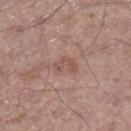Automated image analysis of the tile measured a lesion area of about 4 mm², an eccentricity of roughly 0.8, and two-axis asymmetry of about 0.4. It also reported a border-irregularity index near 5/10, a color-variation rating of about 0.5/10, and peripheral color asymmetry of about 0. It also reported a nevus-likeness score of about 0/100 and lesion-presence confidence of about 100/100. A male patient aged 53–57. The lesion is located on the right lower leg. A region of skin cropped from a whole-body photographic capture, roughly 15 mm wide.